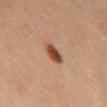biopsy_status: not biopsied; imaged during a skin examination
image:
  source: total-body photography crop
  field_of_view_mm: 15
automated_metrics:
  eccentricity: 0.75
  cielab_L: 45
  cielab_a: 23
  cielab_b: 32
  vs_skin_darker_L: 15.0
  vs_skin_contrast_norm: 11.0
  color_variation_0_10: 4.5
  peripheral_color_asymmetry: 1.5
  nevus_likeness_0_100: 100
  lesion_detection_confidence_0_100: 100
patient:
  sex: male
  age_approx: 65
lesion_size:
  long_diameter_mm_approx: 3.0
site: mid back